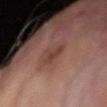{
  "biopsy_status": "not biopsied; imaged during a skin examination",
  "lighting": "cross-polarized",
  "patient": {
    "sex": "female",
    "age_approx": 80
  },
  "lesion_size": {
    "long_diameter_mm_approx": 3.0
  },
  "site": "right arm",
  "automated_metrics": {
    "area_mm2_approx": 4.0,
    "eccentricity": 0.75,
    "shape_asymmetry": 0.4,
    "border_irregularity_0_10": 4.0,
    "color_variation_0_10": 2.0,
    "nevus_likeness_0_100": 15,
    "lesion_detection_confidence_0_100": 100
  },
  "image": {
    "source": "total-body photography crop",
    "field_of_view_mm": 15
  }
}